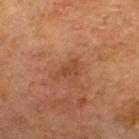| feature | finding |
|---|---|
| workup | no biopsy performed (imaged during a skin exam) |
| subject | male, aged 68 to 72 |
| location | the upper back |
| imaging modality | ~15 mm tile from a whole-body skin photo |
| automated lesion analysis | border irregularity of about 5.5 on a 0–10 scale, a color-variation rating of about 0/10, and peripheral color asymmetry of about 0 |
| lighting | cross-polarized |
| size | about 3 mm |